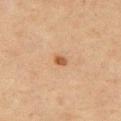Captured during whole-body skin photography for melanoma surveillance; the lesion was not biopsied. This is a cross-polarized tile. A female subject, in their 40s. A 15 mm crop from a total-body photograph taken for skin-cancer surveillance. The lesion is located on the right upper arm. Approximately 1.5 mm at its widest. The lesion-visualizer software estimated a lesion area of about 2 mm², an outline eccentricity of about 0.55 (0 = round, 1 = elongated), and a shape-asymmetry score of about 0.25 (0 = symmetric). The analysis additionally found border irregularity of about 2 on a 0–10 scale and internal color variation of about 1.5 on a 0–10 scale.